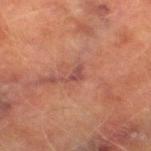Recorded during total-body skin imaging; not selected for excision or biopsy.
The subject is a male aged approximately 75.
The tile uses cross-polarized illumination.
From the leg.
The total-body-photography lesion software estimated a lesion area of about 2.5 mm², a shape eccentricity near 0.85, and two-axis asymmetry of about 0.55.
The lesion's longest dimension is about 3 mm.
This image is a 15 mm lesion crop taken from a total-body photograph.